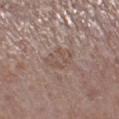Impression:
Imaged during a routine full-body skin examination; the lesion was not biopsied and no histopathology is available.
Context:
The tile uses white-light illumination. Longest diameter approximately 3.5 mm. A 15 mm close-up extracted from a 3D total-body photography capture. A male subject in their mid-70s. The lesion is on the left lower leg.